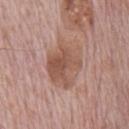Acquisition and patient details:
A male subject, in their 70s. The tile uses white-light illumination. The recorded lesion diameter is about 6 mm. A region of skin cropped from a whole-body photographic capture, roughly 15 mm wide. Located on the chest.